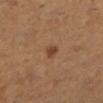This lesion was catalogued during total-body skin photography and was not selected for biopsy.
The lesion is located on the left lower leg.
The tile uses cross-polarized illumination.
A female subject in their mid-50s.
A lesion tile, about 15 mm wide, cut from a 3D total-body photograph.
Longest diameter approximately 1.5 mm.